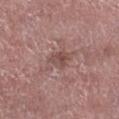Recorded during total-body skin imaging; not selected for excision or biopsy. Longest diameter approximately 3 mm. The lesion-visualizer software estimated a normalized border contrast of about 6.5. The analysis additionally found a border-irregularity rating of about 3/10, internal color variation of about 3.5 on a 0–10 scale, and radial color variation of about 1.5. And it measured an automated nevus-likeness rating near 0 out of 100 and a detector confidence of about 100 out of 100 that the crop contains a lesion. Located on the right lower leg. The subject is a male in their mid- to late 70s. Imaged with white-light lighting. A 15 mm crop from a total-body photograph taken for skin-cancer surveillance.